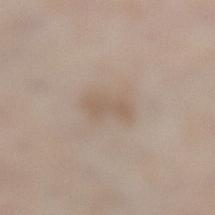workup: catalogued during a skin exam; not biopsied
lighting: white-light
body site: the leg
automated lesion analysis: an area of roughly 5 mm² and two-axis asymmetry of about 0.25; a lesion color around L≈58 a*≈13 b*≈26 in CIELAB, roughly 7 lightness units darker than nearby skin, and a normalized lesion–skin contrast near 5; internal color variation of about 1 on a 0–10 scale and radial color variation of about 0.5
image: ~15 mm crop, total-body skin-cancer survey
diameter: about 3.5 mm
subject: male, in their 60s